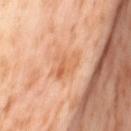Clinical impression: Imaged during a routine full-body skin examination; the lesion was not biopsied and no histopathology is available. Background: Located on the left thigh. This image is a 15 mm lesion crop taken from a total-body photograph. The subject is a female in their mid- to late 50s. Captured under cross-polarized illumination.